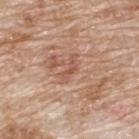<record>
<biopsy_status>not biopsied; imaged during a skin examination</biopsy_status>
<patient>
  <sex>male</sex>
  <age_approx>80</age_approx>
</patient>
<lighting>white-light</lighting>
<site>upper back</site>
<automated_metrics>
  <eccentricity>0.9</eccentricity>
  <shape_asymmetry>0.5</shape_asymmetry>
  <nevus_likeness_0_100>0</nevus_likeness_0_100>
  <lesion_detection_confidence_0_100>90</lesion_detection_confidence_0_100>
</automated_metrics>
<image>
  <source>total-body photography crop</source>
  <field_of_view_mm>15</field_of_view_mm>
</image>
<lesion_size>
  <long_diameter_mm_approx>3.5</long_diameter_mm_approx>
</lesion_size>
</record>